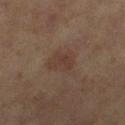Impression: Recorded during total-body skin imaging; not selected for excision or biopsy. Background: A female patient, roughly 60 years of age. The lesion is on the left thigh. A 15 mm crop from a total-body photograph taken for skin-cancer surveillance. Captured under cross-polarized illumination.